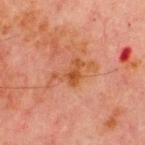No biopsy was performed on this lesion — it was imaged during a full skin examination and was not determined to be concerning. A male patient, aged around 70. Automated tile analysis of the lesion measured an area of roughly 7.5 mm² and an eccentricity of roughly 0.8. And it measured a border-irregularity index near 8.5/10, a color-variation rating of about 3.5/10, and a peripheral color-asymmetry measure near 1. The lesion is located on the chest. Approximately 5 mm at its widest. The tile uses cross-polarized illumination. Cropped from a whole-body photographic skin survey; the tile spans about 15 mm.